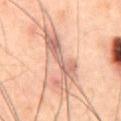Impression:
Captured during whole-body skin photography for melanoma surveillance; the lesion was not biopsied.
Acquisition and patient details:
The lesion is located on the abdomen. The tile uses cross-polarized illumination. A male subject aged approximately 60. A roughly 15 mm field-of-view crop from a total-body skin photograph.